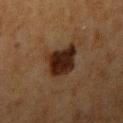Recorded during total-body skin imaging; not selected for excision or biopsy. A 15 mm close-up tile from a total-body photography series done for melanoma screening. Captured under cross-polarized illumination. Longest diameter approximately 4.5 mm. The lesion is on the right upper arm. An algorithmic analysis of the crop reported about 12 CIELAB-L* units darker than the surrounding skin and a normalized lesion–skin contrast near 13. And it measured a border-irregularity index near 3/10, internal color variation of about 4 on a 0–10 scale, and peripheral color asymmetry of about 1. The software also gave a nevus-likeness score of about 85/100 and a lesion-detection confidence of about 100/100. A female patient aged 53 to 57.